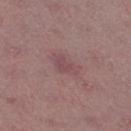| feature | finding |
|---|---|
| notes | imaged on a skin check; not biopsied |
| imaging modality | ~15 mm tile from a whole-body skin photo |
| anatomic site | the right thigh |
| lesion size | ≈3.5 mm |
| subject | female, aged around 45 |
| TBP lesion metrics | a mean CIELAB color near L≈48 a*≈21 b*≈17 and roughly 6 lightness units darker than nearby skin; border irregularity of about 3 on a 0–10 scale, a color-variation rating of about 2/10, and radial color variation of about 0.5; an automated nevus-likeness rating near 0 out of 100 and a detector confidence of about 100 out of 100 that the crop contains a lesion |